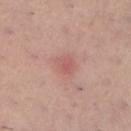workup=imaged on a skin check; not biopsied
size=≈2.5 mm
location=the right lower leg
imaging modality=total-body-photography crop, ~15 mm field of view
subject=female, roughly 60 years of age